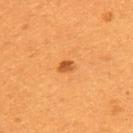The lesion was tiled from a total-body skin photograph and was not biopsied. The lesion is located on the upper back. Automated tile analysis of the lesion measured border irregularity of about 1.5 on a 0–10 scale, a within-lesion color-variation index near 2.5/10, and radial color variation of about 1. A region of skin cropped from a whole-body photographic capture, roughly 15 mm wide. Imaged with cross-polarized lighting. Measured at roughly 2 mm in maximum diameter. A female patient, in their mid-30s.